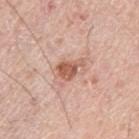Imaged during a routine full-body skin examination; the lesion was not biopsied and no histopathology is available.
On the left thigh.
A 15 mm crop from a total-body photograph taken for skin-cancer surveillance.
A male subject, aged 78 to 82.
The lesion's longest dimension is about 3.5 mm.